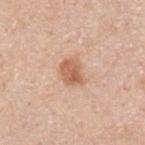workup — imaged on a skin check; not biopsied
site — the upper back
subject — male, aged 28 to 32
image — 15 mm crop, total-body photography
lesion size — about 3.5 mm